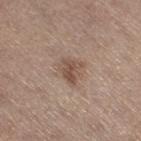Q: Was this lesion biopsied?
A: imaged on a skin check; not biopsied
Q: Automated lesion metrics?
A: a normalized lesion–skin contrast near 7.5; a border-irregularity rating of about 4.5/10 and peripheral color asymmetry of about 1
Q: What are the patient's age and sex?
A: female, approximately 65 years of age
Q: What is the anatomic site?
A: the right thigh
Q: How large is the lesion?
A: ~2.5 mm (longest diameter)
Q: How was the tile lit?
A: white-light
Q: What kind of image is this?
A: total-body-photography crop, ~15 mm field of view